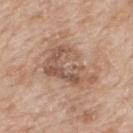{
  "biopsy_status": "not biopsied; imaged during a skin examination",
  "site": "mid back",
  "lighting": "white-light",
  "patient": {
    "sex": "male",
    "age_approx": 65
  },
  "automated_metrics": {
    "area_mm2_approx": 17.0,
    "eccentricity": 0.8,
    "shape_asymmetry": 0.45,
    "cielab_L": 55,
    "cielab_a": 18,
    "cielab_b": 28,
    "vs_skin_darker_L": 10.0,
    "vs_skin_contrast_norm": 7.0,
    "border_irregularity_0_10": 7.5,
    "color_variation_0_10": 5.5
  },
  "lesion_size": {
    "long_diameter_mm_approx": 6.5
  },
  "image": {
    "source": "total-body photography crop",
    "field_of_view_mm": 15
  }
}